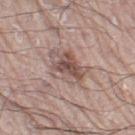– notes: imaged on a skin check; not biopsied